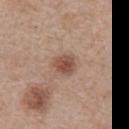Part of a total-body skin-imaging series; this lesion was reviewed on a skin check and was not flagged for biopsy. Approximately 3 mm at its widest. The subject is a male aged around 65. This image is a 15 mm lesion crop taken from a total-body photograph. On the chest. An algorithmic analysis of the crop reported a footprint of about 6 mm², an eccentricity of roughly 0.45, and two-axis asymmetry of about 0.2. The software also gave a lesion color around L≈50 a*≈20 b*≈27 in CIELAB and a lesion-to-skin contrast of about 8 (normalized; higher = more distinct). The software also gave a border-irregularity rating of about 2/10, internal color variation of about 3 on a 0–10 scale, and a peripheral color-asymmetry measure near 1.